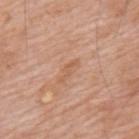Q: Is there a histopathology result?
A: imaged on a skin check; not biopsied
Q: How large is the lesion?
A: ≈2.5 mm
Q: What is the imaging modality?
A: ~15 mm crop, total-body skin-cancer survey
Q: What lighting was used for the tile?
A: white-light illumination
Q: What is the anatomic site?
A: the mid back
Q: Who is the patient?
A: male, approximately 60 years of age